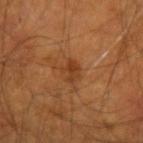This lesion was catalogued during total-body skin photography and was not selected for biopsy. On the left forearm. Cropped from a whole-body photographic skin survey; the tile spans about 15 mm. A male subject roughly 55 years of age. The lesion-visualizer software estimated an area of roughly 4 mm² and a symmetry-axis asymmetry near 0.4. The analysis additionally found a mean CIELAB color near L≈36 a*≈22 b*≈34 and roughly 7 lightness units darker than nearby skin. The analysis additionally found a nevus-likeness score of about 5/100 and lesion-presence confidence of about 100/100.